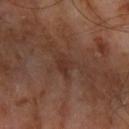{"biopsy_status": "not biopsied; imaged during a skin examination", "lesion_size": {"long_diameter_mm_approx": 3.5}, "image": {"source": "total-body photography crop", "field_of_view_mm": 15}, "lighting": "cross-polarized", "patient": {"sex": "male", "age_approx": 65}, "site": "left forearm"}